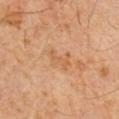{
  "biopsy_status": "not biopsied; imaged during a skin examination",
  "lesion_size": {
    "long_diameter_mm_approx": 3.5
  },
  "lighting": "cross-polarized",
  "patient": {
    "sex": "male",
    "age_approx": 60
  },
  "site": "chest",
  "image": {
    "source": "total-body photography crop",
    "field_of_view_mm": 15
  }
}